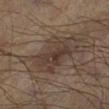  biopsy_status: not biopsied; imaged during a skin examination
  lesion_size:
    long_diameter_mm_approx: 6.0
  lighting: cross-polarized
  image:
    source: total-body photography crop
    field_of_view_mm: 15
  site: left lower leg
  patient:
    sex: male
    age_approx: 55